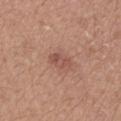- automated metrics — about 8 CIELAB-L* units darker than the surrounding skin
- patient — male, aged 18 to 22
- lighting — white-light
- lesion size — ~2.5 mm (longest diameter)
- anatomic site — the head or neck
- image source — total-body-photography crop, ~15 mm field of view